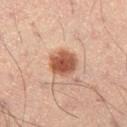Notes:
– notes · no biopsy performed (imaged during a skin exam)
– subject · male, aged 48 to 52
– lesion diameter · about 3.5 mm
– imaging modality · ~15 mm crop, total-body skin-cancer survey
– automated metrics · a border-irregularity index near 1/10, internal color variation of about 3.5 on a 0–10 scale, and radial color variation of about 1; a classifier nevus-likeness of about 100/100 and a detector confidence of about 100 out of 100 that the crop contains a lesion
– tile lighting · cross-polarized
– location · the left thigh